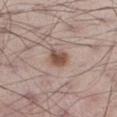Clinical impression: Part of a total-body skin-imaging series; this lesion was reviewed on a skin check and was not flagged for biopsy. Acquisition and patient details: A male subject, roughly 70 years of age. On the left thigh. A 15 mm close-up extracted from a 3D total-body photography capture.